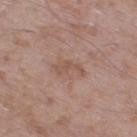Notes:
– biopsy status: imaged on a skin check; not biopsied
– illumination: white-light illumination
– image source: ~15 mm crop, total-body skin-cancer survey
– automated lesion analysis: an area of roughly 4 mm², an outline eccentricity of about 0.9 (0 = round, 1 = elongated), and a shape-asymmetry score of about 0.55 (0 = symmetric); a lesion color around L≈53 a*≈19 b*≈26 in CIELAB and about 7 CIELAB-L* units darker than the surrounding skin; a nevus-likeness score of about 0/100 and a detector confidence of about 100 out of 100 that the crop contains a lesion
– subject: male, about 60 years old
– anatomic site: the left thigh